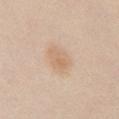Clinical impression: Recorded during total-body skin imaging; not selected for excision or biopsy. Clinical summary: About 4 mm across. A female patient, about 20 years old. Imaged with white-light lighting. A 15 mm crop from a total-body photograph taken for skin-cancer surveillance. The lesion is on the chest. The lesion-visualizer software estimated border irregularity of about 1.5 on a 0–10 scale and internal color variation of about 3 on a 0–10 scale. The software also gave a nevus-likeness score of about 45/100 and a lesion-detection confidence of about 100/100.